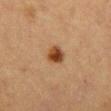notes=no biopsy performed (imaged during a skin exam)
subject=female, in their mid- to late 50s
body site=the abdomen
acquisition=total-body-photography crop, ~15 mm field of view